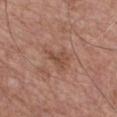• notes · no biopsy performed (imaged during a skin exam)
• TBP lesion metrics · an average lesion color of about L≈47 a*≈22 b*≈29 (CIELAB), roughly 7 lightness units darker than nearby skin, and a normalized border contrast of about 6; a border-irregularity index near 5/10, a within-lesion color-variation index near 1.5/10, and peripheral color asymmetry of about 0.5; a classifier nevus-likeness of about 0/100 and lesion-presence confidence of about 100/100
• anatomic site · the chest
• illumination · white-light illumination
• patient · male, aged 63–67
• lesion diameter · ≈2.5 mm
• image source · total-body-photography crop, ~15 mm field of view